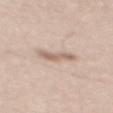The lesion was tiled from a total-body skin photograph and was not biopsied.
The lesion's longest dimension is about 3.5 mm.
The subject is a male approximately 45 years of age.
Located on the mid back.
The tile uses white-light illumination.
A 15 mm crop from a total-body photograph taken for skin-cancer surveillance.